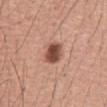{
  "biopsy_status": "not biopsied; imaged during a skin examination",
  "automated_metrics": {
    "area_mm2_approx": 6.5,
    "eccentricity": 0.4,
    "nevus_likeness_0_100": 90
  },
  "lighting": "white-light",
  "lesion_size": {
    "long_diameter_mm_approx": 3.0
  },
  "site": "back",
  "image": {
    "source": "total-body photography crop",
    "field_of_view_mm": 15
  },
  "patient": {
    "sex": "male",
    "age_approx": 45
  }
}